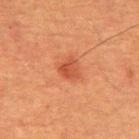notes = catalogued during a skin exam; not biopsied
site = the upper back
lighting = cross-polarized illumination
image source = 15 mm crop, total-body photography
patient = male, about 50 years old